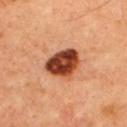This lesion was catalogued during total-body skin photography and was not selected for biopsy. The lesion-visualizer software estimated a footprint of about 12 mm² and a shape eccentricity near 0.6. And it measured about 23 CIELAB-L* units darker than the surrounding skin and a normalized border contrast of about 15. The software also gave internal color variation of about 8 on a 0–10 scale. The analysis additionally found a nevus-likeness score of about 90/100 and a lesion-detection confidence of about 100/100. Imaged with cross-polarized lighting. The lesion's longest dimension is about 4 mm. Located on the upper back. A male subject, aged 53 to 57. A roughly 15 mm field-of-view crop from a total-body skin photograph.